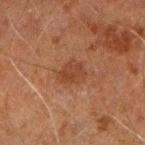Clinical impression:
The lesion was tiled from a total-body skin photograph and was not biopsied.
Acquisition and patient details:
A close-up tile cropped from a whole-body skin photograph, about 15 mm across. Located on the left arm. A male patient aged 58 to 62.